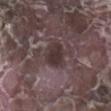Captured during whole-body skin photography for melanoma surveillance; the lesion was not biopsied. A roughly 15 mm field-of-view crop from a total-body skin photograph. The recorded lesion diameter is about 3.5 mm. A male subject roughly 75 years of age. The lesion is located on the right lower leg. Automated image analysis of the tile measured an outline eccentricity of about 0.65 (0 = round, 1 = elongated) and a symmetry-axis asymmetry near 0.25. And it measured an average lesion color of about L≈32 a*≈13 b*≈13 (CIELAB), roughly 9 lightness units darker than nearby skin, and a lesion-to-skin contrast of about 8.5 (normalized; higher = more distinct). The software also gave a classifier nevus-likeness of about 0/100.